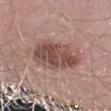{"biopsy_status": "not biopsied; imaged during a skin examination", "patient": {"sex": "male", "age_approx": 50}, "lighting": "white-light", "image": {"source": "total-body photography crop", "field_of_view_mm": 15}, "lesion_size": {"long_diameter_mm_approx": 5.5}, "site": "right forearm", "automated_metrics": {"nevus_likeness_0_100": 15, "lesion_detection_confidence_0_100": 100}}